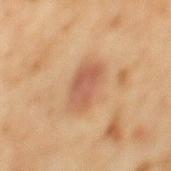Image and clinical context:
Located on the mid back. A male patient, about 60 years old. A 15 mm crop from a total-body photograph taken for skin-cancer surveillance.